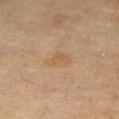Impression: Part of a total-body skin-imaging series; this lesion was reviewed on a skin check and was not flagged for biopsy. Clinical summary: Located on the leg. Longest diameter approximately 3 mm. The patient is a female aged 58 to 62. Captured under cross-polarized illumination. Automated tile analysis of the lesion measured roughly 5 lightness units darker than nearby skin and a lesion-to-skin contrast of about 5 (normalized; higher = more distinct). And it measured a border-irregularity rating of about 4/10 and a color-variation rating of about 1/10. It also reported an automated nevus-likeness rating near 0 out of 100 and a detector confidence of about 100 out of 100 that the crop contains a lesion. A 15 mm crop from a total-body photograph taken for skin-cancer surveillance.